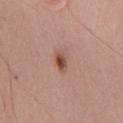Assessment: Part of a total-body skin-imaging series; this lesion was reviewed on a skin check and was not flagged for biopsy. Acquisition and patient details: A male patient aged 63–67. Located on the front of the torso. Automated tile analysis of the lesion measured a footprint of about 3.5 mm², an eccentricity of roughly 0.85, and a shape-asymmetry score of about 0.25 (0 = symmetric). The analysis additionally found a nevus-likeness score of about 100/100 and a lesion-detection confidence of about 100/100. Cropped from a whole-body photographic skin survey; the tile spans about 15 mm.